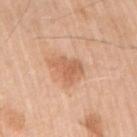| key | value |
|---|---|
| body site | the right upper arm |
| lesion size | ~4 mm (longest diameter) |
| subject | male, aged approximately 80 |
| acquisition | ~15 mm crop, total-body skin-cancer survey |
| lighting | white-light |